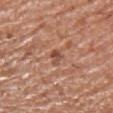Q: Was a biopsy performed?
A: imaged on a skin check; not biopsied
Q: How was the tile lit?
A: white-light
Q: What is the anatomic site?
A: the left upper arm
Q: Lesion size?
A: ~3 mm (longest diameter)
Q: What kind of image is this?
A: ~15 mm tile from a whole-body skin photo
Q: Patient demographics?
A: male, roughly 65 years of age